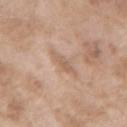| key | value |
|---|---|
| follow-up | catalogued during a skin exam; not biopsied |
| lesion diameter | ≈3 mm |
| image source | ~15 mm crop, total-body skin-cancer survey |
| body site | the left forearm |
| patient | female, in their mid-70s |